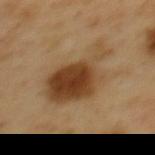Q: Was this lesion biopsied?
A: imaged on a skin check; not biopsied
Q: What are the patient's age and sex?
A: male, roughly 50 years of age
Q: What kind of image is this?
A: ~15 mm tile from a whole-body skin photo
Q: Where on the body is the lesion?
A: the mid back